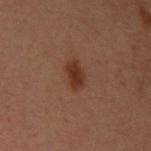No biopsy was performed on this lesion — it was imaged during a full skin examination and was not determined to be concerning.
Captured under cross-polarized illumination.
A lesion tile, about 15 mm wide, cut from a 3D total-body photograph.
On the left upper arm.
A male patient, in their mid-30s.
About 3 mm across.